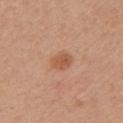The tile uses white-light illumination. A 15 mm close-up extracted from a 3D total-body photography capture. On the left upper arm. A female subject in their mid- to late 40s. Longest diameter approximately 3 mm.